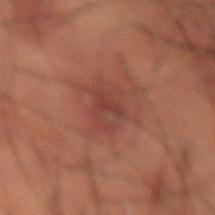Findings:
• notes — total-body-photography surveillance lesion; no biopsy
• diameter — about 4 mm
• patient — male, in their 50s
• location — the lower back
• automated metrics — an area of roughly 7.5 mm², an outline eccentricity of about 0.7 (0 = round, 1 = elongated), and a symmetry-axis asymmetry near 0.3; a mean CIELAB color near L≈32 a*≈21 b*≈21, roughly 6 lightness units darker than nearby skin, and a normalized lesion–skin contrast near 6; an automated nevus-likeness rating near 5 out of 100 and a lesion-detection confidence of about 100/100
• image — total-body-photography crop, ~15 mm field of view
• lighting — cross-polarized illumination